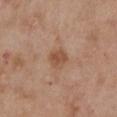<lesion>
<automated_metrics>
  <area_mm2_approx>5.0</area_mm2_approx>
  <eccentricity>0.4</eccentricity>
  <shape_asymmetry>0.25</shape_asymmetry>
  <vs_skin_darker_L>9.0</vs_skin_darker_L>
  <border_irregularity_0_10>2.0</border_irregularity_0_10>
  <color_variation_0_10>2.5</color_variation_0_10>
  <peripheral_color_asymmetry>1.0</peripheral_color_asymmetry>
  <nevus_likeness_0_100>10</nevus_likeness_0_100>
  <lesion_detection_confidence_0_100>100</lesion_detection_confidence_0_100>
</automated_metrics>
<lesion_size>
  <long_diameter_mm_approx>2.5</long_diameter_mm_approx>
</lesion_size>
<lighting>white-light</lighting>
<image>
  <source>total-body photography crop</source>
  <field_of_view_mm>15</field_of_view_mm>
</image>
<patient>
  <sex>female</sex>
  <age_approx>65</age_approx>
</patient>
<site>chest</site>
</lesion>